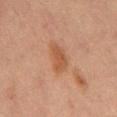Captured during whole-body skin photography for melanoma surveillance; the lesion was not biopsied. Longest diameter approximately 4 mm. The patient is a male aged 63 to 67. Located on the abdomen. Imaged with cross-polarized lighting. An algorithmic analysis of the crop reported a shape eccentricity near 0.85. The analysis additionally found internal color variation of about 2 on a 0–10 scale and peripheral color asymmetry of about 0.5. A 15 mm close-up extracted from a 3D total-body photography capture.